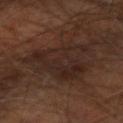notes: imaged on a skin check; not biopsied | patient: male, aged around 60 | image source: 15 mm crop, total-body photography | automated metrics: an average lesion color of about L≈23 a*≈15 b*≈20 (CIELAB), roughly 5 lightness units darker than nearby skin, and a normalized lesion–skin contrast near 6; a border-irregularity index near 5.5/10 and a peripheral color-asymmetry measure near 1 | body site: the arm | lesion diameter: about 7 mm | lighting: cross-polarized illumination.